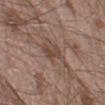biopsy status: catalogued during a skin exam; not biopsied
lighting: white-light illumination
acquisition: 15 mm crop, total-body photography
subject: male, aged 18 to 22
location: the left lower leg